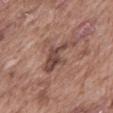No biopsy was performed on this lesion — it was imaged during a full skin examination and was not determined to be concerning.
Approximately 5 mm at its widest.
Automated image analysis of the tile measured a lesion area of about 8.5 mm², an eccentricity of roughly 0.85, and a shape-asymmetry score of about 0.45 (0 = symmetric).
A region of skin cropped from a whole-body photographic capture, roughly 15 mm wide.
A male patient in their mid-70s.
Located on the abdomen.